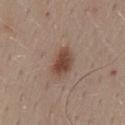Notes:
– workup — total-body-photography surveillance lesion; no biopsy
– subject — female, in their mid-40s
– image source — ~15 mm crop, total-body skin-cancer survey
– site — the back
– lesion size — ~3.5 mm (longest diameter)
– lighting — white-light